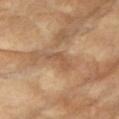Notes:
– anatomic site: the left upper arm
– diameter: about 3.5 mm
– image: 15 mm crop, total-body photography
– patient: female, about 75 years old
– illumination: cross-polarized